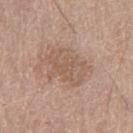image source — ~15 mm crop, total-body skin-cancer survey; patient — male, in their mid- to late 60s; anatomic site — the left thigh.